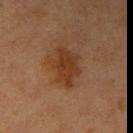| feature | finding |
|---|---|
| anatomic site | the right upper arm |
| image source | ~15 mm crop, total-body skin-cancer survey |
| subject | female, about 40 years old |